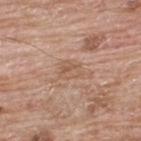biopsy status: total-body-photography surveillance lesion; no biopsy | image source: 15 mm crop, total-body photography | location: the upper back | image-analysis metrics: an area of roughly 4.5 mm² and a symmetry-axis asymmetry near 0.35; a lesion color around L≈56 a*≈19 b*≈30 in CIELAB, a lesion–skin lightness drop of about 7, and a normalized lesion–skin contrast near 5 | subject: male, in their mid- to late 60s | lighting: white-light.